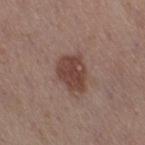Impression: Recorded during total-body skin imaging; not selected for excision or biopsy. Clinical summary: From the right thigh. The patient is a female roughly 40 years of age. The lesion-visualizer software estimated a mean CIELAB color near L≈43 a*≈20 b*≈23, a lesion–skin lightness drop of about 11, and a normalized border contrast of about 8.5. And it measured a border-irregularity rating of about 2/10, a within-lesion color-variation index near 2.5/10, and peripheral color asymmetry of about 1. The analysis additionally found an automated nevus-likeness rating near 80 out of 100 and a detector confidence of about 100 out of 100 that the crop contains a lesion. About 4.5 mm across. The tile uses white-light illumination. A 15 mm close-up tile from a total-body photography series done for melanoma screening.